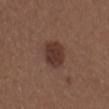From the arm. Cropped from a total-body skin-imaging series; the visible field is about 15 mm. A male patient aged approximately 30.